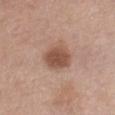Part of a total-body skin-imaging series; this lesion was reviewed on a skin check and was not flagged for biopsy. From the chest. Approximately 3.5 mm at its widest. This is a white-light tile. The subject is a female aged 53 to 57. The total-body-photography lesion software estimated a border-irregularity rating of about 1.5/10, a within-lesion color-variation index near 2.5/10, and a peripheral color-asymmetry measure near 0.5. It also reported an automated nevus-likeness rating near 95 out of 100 and lesion-presence confidence of about 100/100. A 15 mm close-up tile from a total-body photography series done for melanoma screening.